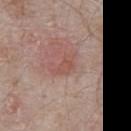Findings:
* workup · total-body-photography surveillance lesion; no biopsy
* lighting · white-light
* imaging modality · ~15 mm tile from a whole-body skin photo
* size · about 3.5 mm
* subject · male, roughly 65 years of age
* location · the chest